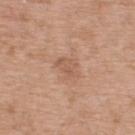Findings:
* biopsy status — no biopsy performed (imaged during a skin exam)
* lighting — white-light
* image — total-body-photography crop, ~15 mm field of view
* body site — the upper back
* automated metrics — a mean CIELAB color near L≈57 a*≈20 b*≈31, about 7 CIELAB-L* units darker than the surrounding skin, and a normalized border contrast of about 5; a border-irregularity rating of about 2.5/10, a within-lesion color-variation index near 2/10, and a peripheral color-asymmetry measure near 0.5
* lesion size — ≈3 mm
* subject — male, aged approximately 55